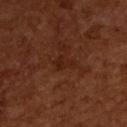workup: total-body-photography surveillance lesion; no biopsy | subject: male, aged approximately 65 | image source: total-body-photography crop, ~15 mm field of view | lighting: cross-polarized.